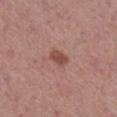follow-up=no biopsy performed (imaged during a skin exam); site=the right lower leg; subject=male, in their 50s; diameter=about 2.5 mm; acquisition=total-body-photography crop, ~15 mm field of view; tile lighting=white-light.